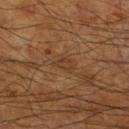Clinical impression: The lesion was photographed on a routine skin check and not biopsied; there is no pathology result. Background: The subject is a male aged 58–62. The lesion-visualizer software estimated a lesion area of about 3 mm², a shape eccentricity near 0.75, and two-axis asymmetry of about 0.45. It also reported a lesion color around L≈37 a*≈18 b*≈31 in CIELAB and a normalized border contrast of about 5. The software also gave a border-irregularity rating of about 4/10, a within-lesion color-variation index near 1.5/10, and radial color variation of about 0.5. The analysis additionally found a classifier nevus-likeness of about 0/100 and a detector confidence of about 95 out of 100 that the crop contains a lesion. A 15 mm close-up tile from a total-body photography series done for melanoma screening. From the leg. Imaged with cross-polarized lighting.